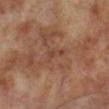notes=catalogued during a skin exam; not biopsied.